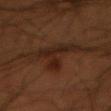The lesion was tiled from a total-body skin photograph and was not biopsied. A male subject aged around 50. A 15 mm close-up tile from a total-body photography series done for melanoma screening. The lesion is located on the arm.